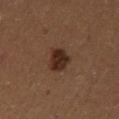Assessment: Part of a total-body skin-imaging series; this lesion was reviewed on a skin check and was not flagged for biopsy. Acquisition and patient details: Cropped from a whole-body photographic skin survey; the tile spans about 15 mm. About 3.5 mm across. Imaged with cross-polarized lighting. The total-body-photography lesion software estimated an outline eccentricity of about 0.75 (0 = round, 1 = elongated) and a shape-asymmetry score of about 0.25 (0 = symmetric). A female patient, roughly 60 years of age. The lesion is located on the left thigh.